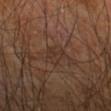Q: Was this lesion biopsied?
A: total-body-photography surveillance lesion; no biopsy
Q: How was the tile lit?
A: cross-polarized illumination
Q: What is the imaging modality?
A: ~15 mm tile from a whole-body skin photo
Q: What did automated image analysis measure?
A: an area of roughly 5.5 mm² and an eccentricity of roughly 0.5; radial color variation of about 1; a classifier nevus-likeness of about 0/100 and lesion-presence confidence of about 70/100
Q: Lesion location?
A: the left upper arm
Q: Lesion size?
A: about 3 mm
Q: What are the patient's age and sex?
A: male, aged 63–67